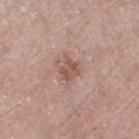{
  "biopsy_status": "not biopsied; imaged during a skin examination",
  "site": "right thigh",
  "lesion_size": {
    "long_diameter_mm_approx": 3.0
  },
  "patient": {
    "sex": "female",
    "age_approx": 75
  },
  "image": {
    "source": "total-body photography crop",
    "field_of_view_mm": 15
  },
  "lighting": "white-light"
}